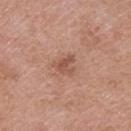follow-up = catalogued during a skin exam; not biopsied
location = the upper back
lesion diameter = about 2.5 mm
image source = total-body-photography crop, ~15 mm field of view
subject = female, in their 40s